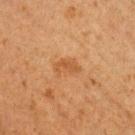biopsy_status: not biopsied; imaged during a skin examination